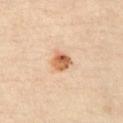Notes:
* notes — total-body-photography surveillance lesion; no biopsy
* site — the right upper arm
* subject — male, aged around 40
* acquisition — 15 mm crop, total-body photography